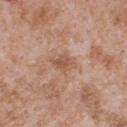biopsy_status: not biopsied; imaged during a skin examination
patient:
  sex: male
  age_approx: 65
image:
  source: total-body photography crop
  field_of_view_mm: 15
site: chest
lighting: white-light
lesion_size:
  long_diameter_mm_approx: 2.5